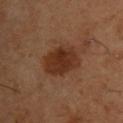Captured during whole-body skin photography for melanoma surveillance; the lesion was not biopsied. Imaged with cross-polarized lighting. A male patient, aged 53–57. The total-body-photography lesion software estimated a border-irregularity index near 1.5/10, a color-variation rating of about 3/10, and peripheral color asymmetry of about 1. A roughly 15 mm field-of-view crop from a total-body skin photograph. From the upper back. The lesion's longest dimension is about 5 mm.